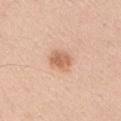{
  "patient": {
    "sex": "male",
    "age_approx": 60
  },
  "site": "right upper arm",
  "automated_metrics": {
    "cielab_L": 64,
    "cielab_a": 22,
    "cielab_b": 34,
    "vs_skin_darker_L": 11.0,
    "vs_skin_contrast_norm": 7.5
  },
  "lesion_size": {
    "long_diameter_mm_approx": 2.5
  },
  "lighting": "white-light",
  "image": {
    "source": "total-body photography crop",
    "field_of_view_mm": 15
  }
}